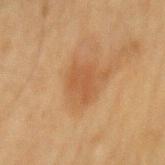Clinical impression:
Part of a total-body skin-imaging series; this lesion was reviewed on a skin check and was not flagged for biopsy.
Context:
A lesion tile, about 15 mm wide, cut from a 3D total-body photograph. The tile uses cross-polarized illumination. A male subject roughly 60 years of age. Measured at roughly 4 mm in maximum diameter. The lesion is on the mid back.